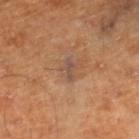workup = no biopsy performed (imaged during a skin exam) | site = the right lower leg | image = ~15 mm tile from a whole-body skin photo | image-analysis metrics = a mean CIELAB color near L≈47 a*≈17 b*≈27 and about 7 CIELAB-L* units darker than the surrounding skin; an automated nevus-likeness rating near 0 out of 100 and a lesion-detection confidence of about 100/100 | size = ≈2.5 mm | patient = male, roughly 60 years of age | tile lighting = cross-polarized illumination.